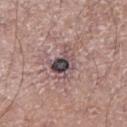No biopsy was performed on this lesion — it was imaged during a full skin examination and was not determined to be concerning. This image is a 15 mm lesion crop taken from a total-body photograph. The lesion is on the right lower leg. The patient is a male in their 70s.